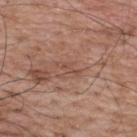The lesion was tiled from a total-body skin photograph and was not biopsied.
The subject is a male in their 70s.
A 15 mm close-up tile from a total-body photography series done for melanoma screening.
Located on the upper back.
The recorded lesion diameter is about 2.5 mm.
Automated tile analysis of the lesion measured a mean CIELAB color near L≈49 a*≈21 b*≈26 and a lesion–skin lightness drop of about 6. The software also gave a border-irregularity index near 4.5/10 and radial color variation of about 0.